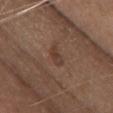image-analysis metrics — a footprint of about 3 mm² and two-axis asymmetry of about 0.4 | acquisition — 15 mm crop, total-body photography | anatomic site — the chest | illumination — white-light illumination | patient — female, about 50 years old.